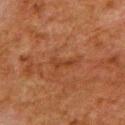The lesion-visualizer software estimated a footprint of about 2.5 mm², an outline eccentricity of about 0.9 (0 = round, 1 = elongated), and a symmetry-axis asymmetry near 0.6. It also reported a nevus-likeness score of about 0/100 and a detector confidence of about 100 out of 100 that the crop contains a lesion.
This is a cross-polarized tile.
From the upper back.
Longest diameter approximately 3 mm.
Cropped from a whole-body photographic skin survey; the tile spans about 15 mm.
A male subject, approximately 80 years of age.